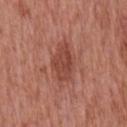<lesion>
<biopsy_status>not biopsied; imaged during a skin examination</biopsy_status>
<image>
  <source>total-body photography crop</source>
  <field_of_view_mm>15</field_of_view_mm>
</image>
<site>front of the torso</site>
<patient>
  <sex>male</sex>
  <age_approx>65</age_approx>
</patient>
<lesion_size>
  <long_diameter_mm_approx>4.5</long_diameter_mm_approx>
</lesion_size>
<lighting>white-light</lighting>
</lesion>